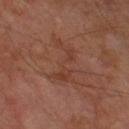Cropped from a total-body skin-imaging series; the visible field is about 15 mm.
Measured at roughly 5.5 mm in maximum diameter.
A male subject, roughly 70 years of age.
The lesion is located on the right thigh.
An algorithmic analysis of the crop reported a mean CIELAB color near L≈38 a*≈22 b*≈28, roughly 5 lightness units darker than nearby skin, and a normalized lesion–skin contrast near 5. The analysis additionally found a border-irregularity index near 9/10, a within-lesion color-variation index near 2/10, and radial color variation of about 0.5. And it measured a classifier nevus-likeness of about 0/100 and a lesion-detection confidence of about 100/100.
Captured under cross-polarized illumination.